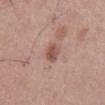notes: total-body-photography surveillance lesion; no biopsy | illumination: white-light illumination | lesion size: ≈3 mm | image source: ~15 mm crop, total-body skin-cancer survey | anatomic site: the mid back | image-analysis metrics: an area of roughly 4 mm² and a shape-asymmetry score of about 0.25 (0 = symmetric); a mean CIELAB color near L≈52 a*≈22 b*≈26, a lesion–skin lightness drop of about 11, and a lesion-to-skin contrast of about 8 (normalized; higher = more distinct); lesion-presence confidence of about 100/100 | patient: male, approximately 55 years of age.